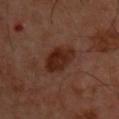No biopsy was performed on this lesion — it was imaged during a full skin examination and was not determined to be concerning.
This image is a 15 mm lesion crop taken from a total-body photograph.
This is a cross-polarized tile.
Located on the upper back.
The lesion-visualizer software estimated an average lesion color of about L≈25 a*≈21 b*≈25 (CIELAB) and roughly 9 lightness units darker than nearby skin. And it measured peripheral color asymmetry of about 1.5.
The subject is a male aged approximately 50.